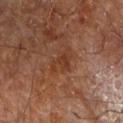{"biopsy_status": "not biopsied; imaged during a skin examination", "image": {"source": "total-body photography crop", "field_of_view_mm": 15}, "site": "arm", "patient": {"sex": "male", "age_approx": "85+"}, "lesion_size": {"long_diameter_mm_approx": 3.0}, "lighting": "cross-polarized", "automated_metrics": {"area_mm2_approx": 4.5, "border_irregularity_0_10": 6.0, "color_variation_0_10": 1.5, "nevus_likeness_0_100": 0, "lesion_detection_confidence_0_100": 100}}